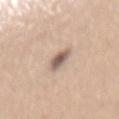workup: imaged on a skin check; not biopsied | imaging modality: total-body-photography crop, ~15 mm field of view | lesion size: about 2.5 mm | anatomic site: the mid back | patient: female, aged 28–32 | illumination: white-light illumination.